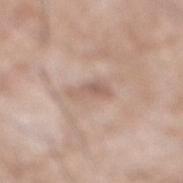This lesion was catalogued during total-body skin photography and was not selected for biopsy. The tile uses white-light illumination. The patient is a male about 60 years old. The total-body-photography lesion software estimated a lesion area of about 5 mm², an outline eccentricity of about 0.85 (0 = round, 1 = elongated), and a symmetry-axis asymmetry near 0.35. The analysis additionally found a lesion-detection confidence of about 100/100. This image is a 15 mm lesion crop taken from a total-body photograph. Measured at roughly 3.5 mm in maximum diameter. The lesion is located on the leg.